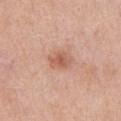Part of a total-body skin-imaging series; this lesion was reviewed on a skin check and was not flagged for biopsy. Cropped from a total-body skin-imaging series; the visible field is about 15 mm. A male patient, approximately 70 years of age. Located on the abdomen.